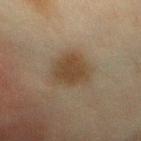workup: no biopsy performed (imaged during a skin exam)
tile lighting: cross-polarized illumination
patient: female, aged around 40
lesion size: ~4 mm (longest diameter)
site: the chest
image-analysis metrics: a shape eccentricity near 0.4; a lesion color around L≈35 a*≈11 b*≈26 in CIELAB, about 8 CIELAB-L* units darker than the surrounding skin, and a normalized border contrast of about 8
image source: ~15 mm crop, total-body skin-cancer survey